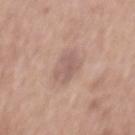{
  "biopsy_status": "not biopsied; imaged during a skin examination",
  "automated_metrics": {
    "area_mm2_approx": 9.0,
    "eccentricity": 0.8,
    "shape_asymmetry": 0.2,
    "border_irregularity_0_10": 2.5,
    "color_variation_0_10": 2.5,
    "peripheral_color_asymmetry": 1.0
  },
  "patient": {
    "sex": "male",
    "age_approx": 60
  },
  "image": {
    "source": "total-body photography crop",
    "field_of_view_mm": 15
  },
  "lesion_size": {
    "long_diameter_mm_approx": 4.5
  },
  "site": "mid back"
}